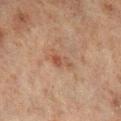notes: total-body-photography surveillance lesion; no biopsy | location: the leg | image source: ~15 mm tile from a whole-body skin photo | subject: male, aged approximately 70.